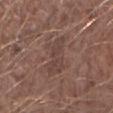The lesion was tiled from a total-body skin photograph and was not biopsied.
An algorithmic analysis of the crop reported border irregularity of about 4.5 on a 0–10 scale, internal color variation of about 2.5 on a 0–10 scale, and a peripheral color-asymmetry measure near 1. The software also gave a classifier nevus-likeness of about 0/100 and a detector confidence of about 95 out of 100 that the crop contains a lesion.
This image is a 15 mm lesion crop taken from a total-body photograph.
A male subject, in their 60s.
This is a white-light tile.
The lesion's longest dimension is about 4.5 mm.
The lesion is located on the right forearm.